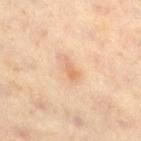- workup: imaged on a skin check; not biopsied
- imaging modality: ~15 mm crop, total-body skin-cancer survey
- location: the right thigh
- illumination: cross-polarized
- patient: male, about 60 years old
- image-analysis metrics: a footprint of about 2.5 mm² and a symmetry-axis asymmetry near 0.35; a lesion color around L≈64 a*≈20 b*≈34 in CIELAB and a normalized border contrast of about 5.5; a nevus-likeness score of about 0/100 and a detector confidence of about 100 out of 100 that the crop contains a lesion
- lesion size: ~2.5 mm (longest diameter)